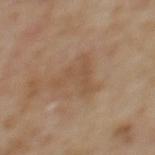Assessment:
Captured during whole-body skin photography for melanoma surveillance; the lesion was not biopsied.
Background:
The patient is a female aged 58 to 62. The recorded lesion diameter is about 5.5 mm. From the upper back. A lesion tile, about 15 mm wide, cut from a 3D total-body photograph.